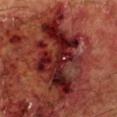subject: male, aged around 60
imaging modality: total-body-photography crop, ~15 mm field of view
site: the left lower leg
TBP lesion metrics: a lesion area of about 50 mm², an eccentricity of roughly 0.8, and two-axis asymmetry of about 0.25; a lesion color around L≈28 a*≈30 b*≈24 in CIELAB, roughly 13 lightness units darker than nearby skin, and a normalized lesion–skin contrast near 12.5; a nevus-likeness score of about 0/100 and a detector confidence of about 90 out of 100 that the crop contains a lesion